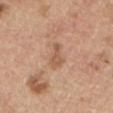Clinical summary:
The lesion is located on the back. The subject is a male roughly 70 years of age. This image is a 15 mm lesion crop taken from a total-body photograph. Longest diameter approximately 3.5 mm. Automated image analysis of the tile measured a footprint of about 5 mm², a shape eccentricity near 0.85, and a shape-asymmetry score of about 0.4 (0 = symmetric). The software also gave an automated nevus-likeness rating near 0 out of 100 and lesion-presence confidence of about 100/100. Imaged with white-light lighting.